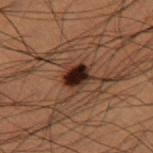No biopsy was performed on this lesion — it was imaged during a full skin examination and was not determined to be concerning. From the right thigh. A 15 mm close-up tile from a total-body photography series done for melanoma screening. Approximately 3.5 mm at its widest. The subject is a male aged 48 to 52. Captured under cross-polarized illumination. Automated tile analysis of the lesion measured a mean CIELAB color near L≈21 a*≈16 b*≈19 and about 13 CIELAB-L* units darker than the surrounding skin. The analysis additionally found border irregularity of about 2 on a 0–10 scale and a color-variation rating of about 7.5/10. The analysis additionally found an automated nevus-likeness rating near 95 out of 100 and lesion-presence confidence of about 100/100.